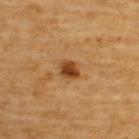Q: Was a biopsy performed?
A: no biopsy performed (imaged during a skin exam)
Q: Patient demographics?
A: male, roughly 60 years of age
Q: What did automated image analysis measure?
A: a lesion area of about 4 mm² and a symmetry-axis asymmetry near 0.2; peripheral color asymmetry of about 1.5; a classifier nevus-likeness of about 90/100
Q: What kind of image is this?
A: ~15 mm crop, total-body skin-cancer survey
Q: How large is the lesion?
A: ~2.5 mm (longest diameter)
Q: Where on the body is the lesion?
A: the upper back
Q: What lighting was used for the tile?
A: cross-polarized illumination